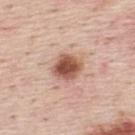Case summary:
* follow-up · imaged on a skin check; not biopsied
* diameter · ~3.5 mm (longest diameter)
* image source · total-body-photography crop, ~15 mm field of view
* site · the upper back
* patient · male, aged 43–47